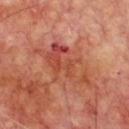Assessment:
Recorded during total-body skin imaging; not selected for excision or biopsy.